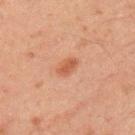notes = imaged on a skin check; not biopsied
acquisition = total-body-photography crop, ~15 mm field of view
TBP lesion metrics = a lesion area of about 3.5 mm² and an eccentricity of roughly 0.8
diameter = ≈2.5 mm
site = the left upper arm
subject = male, aged 43–47
illumination = cross-polarized illumination